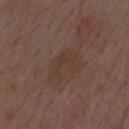Q: Is there a histopathology result?
A: no biopsy performed (imaged during a skin exam)
Q: Lesion location?
A: the mid back
Q: What is the lesion's diameter?
A: ~4.5 mm (longest diameter)
Q: What is the imaging modality?
A: ~15 mm crop, total-body skin-cancer survey
Q: Automated lesion metrics?
A: an area of roughly 8 mm², an eccentricity of roughly 0.75, and a symmetry-axis asymmetry near 0.5; a border-irregularity index near 6.5/10, internal color variation of about 2 on a 0–10 scale, and radial color variation of about 0.5
Q: What are the patient's age and sex?
A: male, roughly 70 years of age
Q: How was the tile lit?
A: white-light